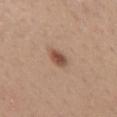Background: From the chest. A female patient in their mid- to late 40s. A 15 mm close-up extracted from a 3D total-body photography capture.